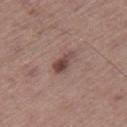No biopsy was performed on this lesion — it was imaged during a full skin examination and was not determined to be concerning.
A 15 mm crop from a total-body photograph taken for skin-cancer surveillance.
The lesion is on the right thigh.
Automated tile analysis of the lesion measured an automated nevus-likeness rating near 80 out of 100.
This is a white-light tile.
A male patient aged approximately 65.
Measured at roughly 3 mm in maximum diameter.